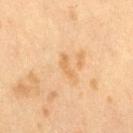Notes:
• workup: catalogued during a skin exam; not biopsied
• image source: total-body-photography crop, ~15 mm field of view
• size: ~3 mm (longest diameter)
• tile lighting: cross-polarized
• patient: female, roughly 40 years of age
• automated lesion analysis: a lesion–skin lightness drop of about 6 and a lesion-to-skin contrast of about 5 (normalized; higher = more distinct); a within-lesion color-variation index near 0/10 and a peripheral color-asymmetry measure near 0; a nevus-likeness score of about 0/100 and lesion-presence confidence of about 100/100
• location: the right thigh